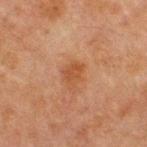A male patient aged approximately 65. A lesion tile, about 15 mm wide, cut from a 3D total-body photograph. The recorded lesion diameter is about 3 mm. Located on the chest.